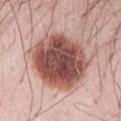Clinical impression: The lesion was photographed on a routine skin check and not biopsied; there is no pathology result. Background: Located on the abdomen. A 15 mm crop from a total-body photograph taken for skin-cancer surveillance. A female subject in their mid- to late 40s.